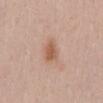| key | value |
|---|---|
| imaging modality | total-body-photography crop, ~15 mm field of view |
| body site | the chest |
| illumination | white-light illumination |
| subject | male, aged 53 to 57 |